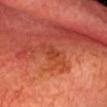Findings:
– notes — imaged on a skin check; not biopsied
– image source — 15 mm crop, total-body photography
– location — the head or neck
– patient — male, approximately 55 years of age